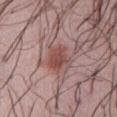Q: Is there a histopathology result?
A: imaged on a skin check; not biopsied
Q: Patient demographics?
A: female, in their 50s
Q: What is the lesion's diameter?
A: about 3.5 mm
Q: What is the imaging modality?
A: total-body-photography crop, ~15 mm field of view
Q: How was the tile lit?
A: white-light illumination
Q: Lesion location?
A: the abdomen